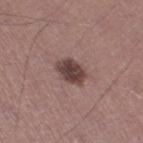workup=catalogued during a skin exam; not biopsied | tile lighting=white-light | anatomic site=the leg | subject=male, roughly 45 years of age | acquisition=~15 mm crop, total-body skin-cancer survey | size=~3.5 mm (longest diameter) | automated metrics=an average lesion color of about L≈41 a*≈18 b*≈19 (CIELAB) and roughly 14 lightness units darker than nearby skin.